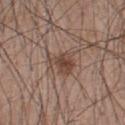No biopsy was performed on this lesion — it was imaged during a full skin examination and was not determined to be concerning. From the upper back. The total-body-photography lesion software estimated a color-variation rating of about 4/10. The software also gave an automated nevus-likeness rating near 65 out of 100 and a lesion-detection confidence of about 100/100. A male patient, aged approximately 50. The lesion's longest dimension is about 4 mm. Cropped from a total-body skin-imaging series; the visible field is about 15 mm. Imaged with white-light lighting.